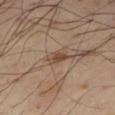Case summary:
• illumination: cross-polarized
• anatomic site: the right thigh
• lesion size: ~3 mm (longest diameter)
• imaging modality: ~15 mm tile from a whole-body skin photo
• patient: male, aged 53–57